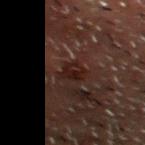Imaged during a routine full-body skin examination; the lesion was not biopsied and no histopathology is available.
A male patient, aged around 60.
A lesion tile, about 15 mm wide, cut from a 3D total-body photograph.
The tile uses cross-polarized illumination.
The lesion's longest dimension is about 2.5 mm.
Located on the head or neck.